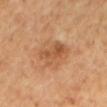location = the mid back | patient = female | image = ~15 mm crop, total-body skin-cancer survey | size = ≈4.5 mm | tile lighting = cross-polarized.